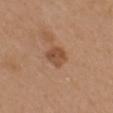location: the right upper arm
subject: female, roughly 40 years of age
imaging modality: ~15 mm crop, total-body skin-cancer survey